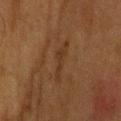workup: imaged on a skin check; not biopsied
image-analysis metrics: border irregularity of about 8 on a 0–10 scale, internal color variation of about 0.5 on a 0–10 scale, and a peripheral color-asymmetry measure near 0; a nevus-likeness score of about 0/100 and a lesion-detection confidence of about 60/100
image: total-body-photography crop, ~15 mm field of view
anatomic site: the head or neck
size: ~5 mm (longest diameter)
patient: male, aged approximately 75
tile lighting: cross-polarized illumination